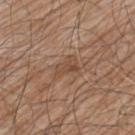Clinical impression: Captured during whole-body skin photography for melanoma surveillance; the lesion was not biopsied. Clinical summary: This is a white-light tile. A male subject in their 80s. About 3 mm across. On the left upper arm. Automated image analysis of the tile measured a classifier nevus-likeness of about 0/100 and a lesion-detection confidence of about 90/100. A 15 mm close-up extracted from a 3D total-body photography capture.